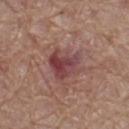Imaged during a routine full-body skin examination; the lesion was not biopsied and no histopathology is available.
This is a white-light tile.
The recorded lesion diameter is about 4 mm.
A 15 mm crop from a total-body photograph taken for skin-cancer surveillance.
On the leg.
The patient is a male roughly 65 years of age.
Automated image analysis of the tile measured an average lesion color of about L≈43 a*≈24 b*≈20 (CIELAB). And it measured a detector confidence of about 100 out of 100 that the crop contains a lesion.